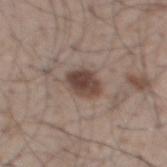Captured during whole-body skin photography for melanoma surveillance; the lesion was not biopsied. A region of skin cropped from a whole-body photographic capture, roughly 15 mm wide. Approximately 3 mm at its widest. A male patient, approximately 50 years of age. This is a white-light tile. An algorithmic analysis of the crop reported an area of roughly 7 mm², an eccentricity of roughly 0.6, and two-axis asymmetry of about 0.15. And it measured a lesion color around L≈43 a*≈16 b*≈22 in CIELAB, about 13 CIELAB-L* units darker than the surrounding skin, and a normalized border contrast of about 10. Located on the back.